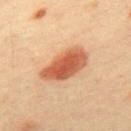| feature | finding |
|---|---|
| workup | imaged on a skin check; not biopsied |
| image-analysis metrics | an eccentricity of roughly 0.85 and two-axis asymmetry of about 0.2; a mean CIELAB color near L≈52 a*≈26 b*≈34, a lesion–skin lightness drop of about 14, and a lesion-to-skin contrast of about 10 (normalized; higher = more distinct); a nevus-likeness score of about 100/100 |
| location | the upper back |
| imaging modality | ~15 mm tile from a whole-body skin photo |
| lesion size | about 6 mm |
| patient | male, aged 38 to 42 |